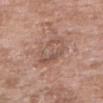Q: Who is the patient?
A: female, aged 68–72
Q: How large is the lesion?
A: about 6 mm
Q: How was this image acquired?
A: total-body-photography crop, ~15 mm field of view
Q: Where on the body is the lesion?
A: the arm
Q: What lighting was used for the tile?
A: white-light illumination
Q: Automated lesion metrics?
A: border irregularity of about 2.5 on a 0–10 scale, a color-variation rating of about 6/10, and a peripheral color-asymmetry measure near 2; a nevus-likeness score of about 0/100 and a detector confidence of about 100 out of 100 that the crop contains a lesion